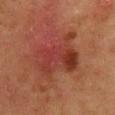<lesion>
  <site>chest</site>
  <image>
    <source>total-body photography crop</source>
    <field_of_view_mm>15</field_of_view_mm>
  </image>
  <patient>
    <sex>female</sex>
    <age_approx>50</age_approx>
  </patient>
</lesion>